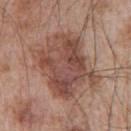Q: Where on the body is the lesion?
A: the left upper arm
Q: How was this image acquired?
A: 15 mm crop, total-body photography
Q: Who is the patient?
A: male, aged around 65
Q: What lighting was used for the tile?
A: white-light illumination
Q: Lesion size?
A: ≈8 mm